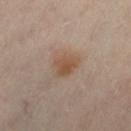• follow-up: catalogued during a skin exam; not biopsied